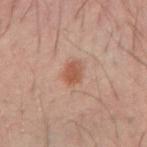Q: Who is the patient?
A: male, roughly 40 years of age
Q: What is the lesion's diameter?
A: ~2.5 mm (longest diameter)
Q: What kind of image is this?
A: ~15 mm tile from a whole-body skin photo
Q: What lighting was used for the tile?
A: cross-polarized illumination
Q: Lesion location?
A: the right forearm